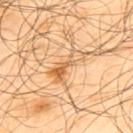follow-up: imaged on a skin check; not biopsied
subject: male, about 65 years old
size: ≈5.5 mm
site: the upper back
image: 15 mm crop, total-body photography
automated metrics: an average lesion color of about L≈62 a*≈20 b*≈40 (CIELAB), roughly 10 lightness units darker than nearby skin, and a lesion-to-skin contrast of about 6.5 (normalized; higher = more distinct); a border-irregularity rating of about 6/10 and peripheral color asymmetry of about 2; a detector confidence of about 95 out of 100 that the crop contains a lesion
illumination: cross-polarized illumination